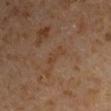Impression: The lesion was photographed on a routine skin check and not biopsied; there is no pathology result. Image and clinical context: The lesion-visualizer software estimated a footprint of about 4 mm² and two-axis asymmetry of about 0.35. The software also gave a nevus-likeness score of about 0/100. Imaged with cross-polarized lighting. From the left upper arm. The recorded lesion diameter is about 3 mm. A male subject, aged 58–62. This image is a 15 mm lesion crop taken from a total-body photograph.